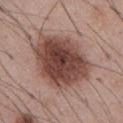Context:
A lesion tile, about 15 mm wide, cut from a 3D total-body photograph. From the front of the torso. The tile uses white-light illumination. Approximately 8 mm at its widest. The subject is a male in their mid-50s. An algorithmic analysis of the crop reported a border-irregularity index near 2/10, internal color variation of about 6 on a 0–10 scale, and peripheral color asymmetry of about 2. The software also gave a nevus-likeness score of about 70/100 and a detector confidence of about 100 out of 100 that the crop contains a lesion.